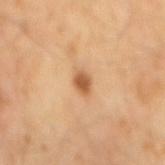The lesion was photographed on a routine skin check and not biopsied; there is no pathology result. A close-up tile cropped from a whole-body skin photograph, about 15 mm across. A male patient, in their mid-50s. Located on the back. This is a cross-polarized tile.